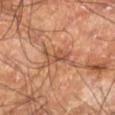{
  "biopsy_status": "not biopsied; imaged during a skin examination",
  "lighting": "cross-polarized",
  "site": "left lower leg",
  "image": {
    "source": "total-body photography crop",
    "field_of_view_mm": 15
  },
  "patient": {
    "sex": "male",
    "age_approx": 60
  }
}